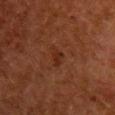  biopsy_status: not biopsied; imaged during a skin examination
  site: right upper arm
  image:
    source: total-body photography crop
    field_of_view_mm: 15
  patient:
    sex: female
    age_approx: 50